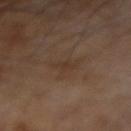Impression:
The lesion was tiled from a total-body skin photograph and was not biopsied.
Clinical summary:
The patient is a male in their 70s. Located on the right forearm. Cropped from a total-body skin-imaging series; the visible field is about 15 mm.